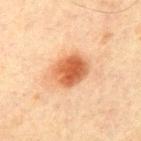No biopsy was performed on this lesion — it was imaged during a full skin examination and was not determined to be concerning. This image is a 15 mm lesion crop taken from a total-body photograph. The lesion is located on the chest. An algorithmic analysis of the crop reported an area of roughly 12 mm², an outline eccentricity of about 0.65 (0 = round, 1 = elongated), and a symmetry-axis asymmetry near 0.1. The analysis additionally found a lesion–skin lightness drop of about 13 and a normalized lesion–skin contrast near 9.5. Approximately 4.5 mm at its widest. Captured under cross-polarized illumination. A male subject, in their 70s.